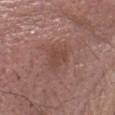This lesion was catalogued during total-body skin photography and was not selected for biopsy. This image is a 15 mm lesion crop taken from a total-body photograph. From the head or neck. A male subject aged around 75. Approximately 2.5 mm at its widest.